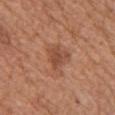Q: Was a biopsy performed?
A: catalogued during a skin exam; not biopsied
Q: How was the tile lit?
A: white-light illumination
Q: Patient demographics?
A: female, aged approximately 55
Q: What is the imaging modality?
A: ~15 mm tile from a whole-body skin photo
Q: What is the lesion's diameter?
A: ~3.5 mm (longest diameter)
Q: Automated lesion metrics?
A: a footprint of about 7 mm², an outline eccentricity of about 0.55 (0 = round, 1 = elongated), and a symmetry-axis asymmetry near 0.35; a border-irregularity rating of about 3.5/10, a color-variation rating of about 3/10, and radial color variation of about 1
Q: What is the anatomic site?
A: the chest